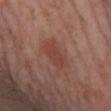Recorded during total-body skin imaging; not selected for excision or biopsy.
The lesion-visualizer software estimated internal color variation of about 2 on a 0–10 scale.
On the left lower leg.
Measured at roughly 3.5 mm in maximum diameter.
Captured under cross-polarized illumination.
This image is a 15 mm lesion crop taken from a total-body photograph.
A female subject, about 50 years old.